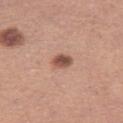<record>
<biopsy_status>not biopsied; imaged during a skin examination</biopsy_status>
<automated_metrics>
  <area_mm2_approx>4.5</area_mm2_approx>
  <shape_asymmetry>0.15</shape_asymmetry>
  <cielab_L>52</cielab_L>
  <cielab_a>22</cielab_a>
  <cielab_b>27</cielab_b>
  <vs_skin_darker_L>14.0</vs_skin_darker_L>
  <vs_skin_contrast_norm>9.0</vs_skin_contrast_norm>
  <border_irregularity_0_10>1.0</border_irregularity_0_10>
  <color_variation_0_10>4.5</color_variation_0_10>
  <peripheral_color_asymmetry>1.5</peripheral_color_asymmetry>
  <nevus_likeness_0_100>95</nevus_likeness_0_100>
</automated_metrics>
<lighting>white-light</lighting>
<site>left thigh</site>
<patient>
  <sex>female</sex>
  <age_approx>30</age_approx>
</patient>
<image>
  <source>total-body photography crop</source>
  <field_of_view_mm>15</field_of_view_mm>
</image>
<lesion_size>
  <long_diameter_mm_approx>2.5</long_diameter_mm_approx>
</lesion_size>
</record>